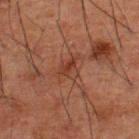| feature | finding |
|---|---|
| follow-up | imaged on a skin check; not biopsied |
| image source | ~15 mm crop, total-body skin-cancer survey |
| site | the upper back |
| subject | male, aged 48 to 52 |
| lighting | cross-polarized |